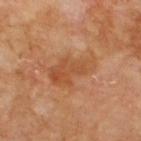The lesion was tiled from a total-body skin photograph and was not biopsied. The total-body-photography lesion software estimated a lesion area of about 13 mm², an outline eccentricity of about 0.85 (0 = round, 1 = elongated), and two-axis asymmetry of about 0.35. It also reported a mean CIELAB color near L≈50 a*≈24 b*≈37 and roughly 7 lightness units darker than nearby skin. The analysis additionally found a peripheral color-asymmetry measure near 1.5. About 5.5 mm across. The patient is a male aged around 70. Cropped from a total-body skin-imaging series; the visible field is about 15 mm. The lesion is on the upper back.